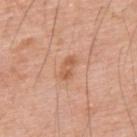follow-up: total-body-photography surveillance lesion; no biopsy | patient: male, in their 60s | tile lighting: white-light illumination | acquisition: ~15 mm crop, total-body skin-cancer survey | lesion diameter: ~3 mm (longest diameter) | anatomic site: the upper back.